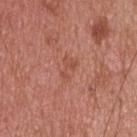{
  "biopsy_status": "not biopsied; imaged during a skin examination",
  "lesion_size": {
    "long_diameter_mm_approx": 3.0
  },
  "patient": {
    "sex": "male",
    "age_approx": 55
  },
  "image": {
    "source": "total-body photography crop",
    "field_of_view_mm": 15
  },
  "site": "upper back",
  "lighting": "white-light",
  "automated_metrics": {
    "area_mm2_approx": 4.5,
    "eccentricity": 0.8,
    "border_irregularity_0_10": 3.5,
    "peripheral_color_asymmetry": 1.5,
    "nevus_likeness_0_100": 0,
    "lesion_detection_confidence_0_100": 100
  }
}